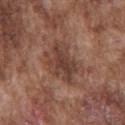Impression: This lesion was catalogued during total-body skin photography and was not selected for biopsy. Background: A 15 mm crop from a total-body photograph taken for skin-cancer surveillance. The recorded lesion diameter is about 5.5 mm. Automated image analysis of the tile measured an area of roughly 12 mm², a shape eccentricity near 0.75, and a shape-asymmetry score of about 0.3 (0 = symmetric). And it measured a border-irregularity index near 4.5/10, a color-variation rating of about 4/10, and radial color variation of about 1.5. It also reported a classifier nevus-likeness of about 0/100. The lesion is on the chest. The patient is a male roughly 75 years of age.